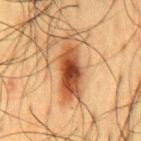Captured under cross-polarized illumination.
A male patient aged approximately 60.
Automated image analysis of the tile measured a lesion area of about 15 mm², a shape eccentricity near 0.95, and a symmetry-axis asymmetry near 0.3. It also reported a lesion color around L≈40 a*≈21 b*≈32 in CIELAB and a normalized lesion–skin contrast near 11.5. It also reported a border-irregularity index near 4.5/10 and peripheral color asymmetry of about 1.5.
A 15 mm close-up extracted from a 3D total-body photography capture.
On the back.